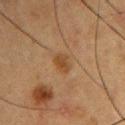notes=total-body-photography surveillance lesion; no biopsy | imaging modality=15 mm crop, total-body photography | tile lighting=cross-polarized illumination | subject=male, aged approximately 55 | site=the right upper arm | size=about 2.5 mm.